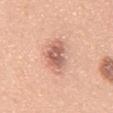biopsy status — no biopsy performed (imaged during a skin exam); illumination — white-light; lesion size — ≈5.5 mm; automated metrics — a nevus-likeness score of about 65/100; patient — male, aged approximately 50; anatomic site — the abdomen; image source — total-body-photography crop, ~15 mm field of view.